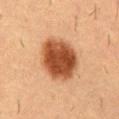| feature | finding |
|---|---|
| notes | total-body-photography surveillance lesion; no biopsy |
| subject | male, approximately 55 years of age |
| lesion size | ~6 mm (longest diameter) |
| tile lighting | cross-polarized |
| location | the mid back |
| image source | ~15 mm crop, total-body skin-cancer survey |